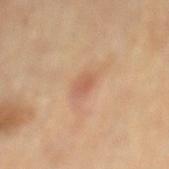Q: Was this lesion biopsied?
A: imaged on a skin check; not biopsied
Q: What kind of image is this?
A: ~15 mm tile from a whole-body skin photo
Q: What is the anatomic site?
A: the mid back
Q: Who is the patient?
A: male, approximately 85 years of age or older